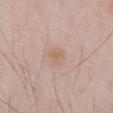The lesion was photographed on a routine skin check and not biopsied; there is no pathology result. On the abdomen. A close-up tile cropped from a whole-body skin photograph, about 15 mm across. The recorded lesion diameter is about 2.5 mm. A male patient aged approximately 55.